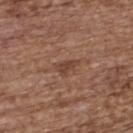Captured during whole-body skin photography for melanoma surveillance; the lesion was not biopsied.
The tile uses white-light illumination.
A close-up tile cropped from a whole-body skin photograph, about 15 mm across.
The recorded lesion diameter is about 2.5 mm.
Located on the upper back.
A female subject about 65 years old.
The lesion-visualizer software estimated a footprint of about 3 mm² and an eccentricity of roughly 0.8. The software also gave a mean CIELAB color near L≈42 a*≈20 b*≈28, about 8 CIELAB-L* units darker than the surrounding skin, and a normalized border contrast of about 6.5.